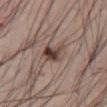biopsy_status: not biopsied; imaged during a skin examination
automated_metrics:
  area_mm2_approx: 6.0
  eccentricity: 0.45
  shape_asymmetry: 0.4
  cielab_L: 42
  cielab_a: 16
  cielab_b: 22
  vs_skin_darker_L: 13.0
  nevus_likeness_0_100: 85
  lesion_detection_confidence_0_100: 100
lighting: white-light
site: abdomen
patient:
  sex: male
  age_approx: 40
image:
  source: total-body photography crop
  field_of_view_mm: 15
lesion_size:
  long_diameter_mm_approx: 3.0